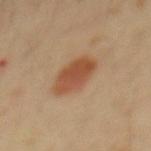Imaged during a routine full-body skin examination; the lesion was not biopsied and no histopathology is available.
This is a cross-polarized tile.
A 15 mm crop from a total-body photograph taken for skin-cancer surveillance.
The lesion is on the mid back.
The lesion-visualizer software estimated a lesion color around L≈48 a*≈22 b*≈33 in CIELAB, roughly 11 lightness units darker than nearby skin, and a lesion-to-skin contrast of about 8.5 (normalized; higher = more distinct). The analysis additionally found border irregularity of about 2 on a 0–10 scale, internal color variation of about 3.5 on a 0–10 scale, and radial color variation of about 1.
The subject is a male roughly 30 years of age.
The lesion's longest dimension is about 5 mm.